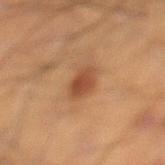• biopsy status — imaged on a skin check; not biopsied
• patient — male, aged approximately 55
• tile lighting — cross-polarized
• image source — ~15 mm crop, total-body skin-cancer survey
• body site — the right lower leg